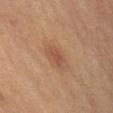Impression:
Captured during whole-body skin photography for melanoma surveillance; the lesion was not biopsied.
Context:
The lesion is on the arm. A female subject aged 63–67. Captured under cross-polarized illumination. A close-up tile cropped from a whole-body skin photograph, about 15 mm across.